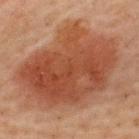Assessment:
No biopsy was performed on this lesion — it was imaged during a full skin examination and was not determined to be concerning.
Acquisition and patient details:
A male patient, aged around 60. This is a cross-polarized tile. Longest diameter approximately 11 mm. Cropped from a total-body skin-imaging series; the visible field is about 15 mm. On the upper back.